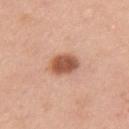Findings:
• follow-up · total-body-photography surveillance lesion; no biopsy
• anatomic site · the left upper arm
• illumination · white-light
• diameter · about 3.5 mm
• acquisition · total-body-photography crop, ~15 mm field of view
• subject · female, in their mid-30s
• image-analysis metrics · border irregularity of about 1 on a 0–10 scale and a peripheral color-asymmetry measure near 1; an automated nevus-likeness rating near 100 out of 100 and a lesion-detection confidence of about 100/100